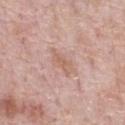Assessment: Recorded during total-body skin imaging; not selected for excision or biopsy. Clinical summary: The lesion is on the chest. The tile uses white-light illumination. A male patient approximately 70 years of age. A close-up tile cropped from a whole-body skin photograph, about 15 mm across.